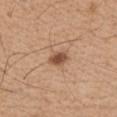Findings:
- workup: no biopsy performed (imaged during a skin exam)
- imaging modality: 15 mm crop, total-body photography
- subject: male, about 65 years old
- location: the front of the torso
- diameter: ≈2.5 mm